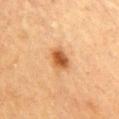The lesion was tiled from a total-body skin photograph and was not biopsied. Measured at roughly 3 mm in maximum diameter. The total-body-photography lesion software estimated a mean CIELAB color near L≈50 a*≈23 b*≈38, about 13 CIELAB-L* units darker than the surrounding skin, and a normalized border contrast of about 9.5. The analysis additionally found a color-variation rating of about 5/10 and a peripheral color-asymmetry measure near 1.5. The analysis additionally found an automated nevus-likeness rating near 95 out of 100 and lesion-presence confidence of about 100/100. A male patient, aged around 85 or older. Imaged with cross-polarized lighting. A 15 mm close-up extracted from a 3D total-body photography capture. The lesion is on the arm.